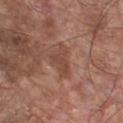This lesion was catalogued during total-body skin photography and was not selected for biopsy. From the chest. Measured at roughly 4 mm in maximum diameter. An algorithmic analysis of the crop reported an average lesion color of about L≈46 a*≈22 b*≈27 (CIELAB), a lesion–skin lightness drop of about 7, and a normalized border contrast of about 6. It also reported an automated nevus-likeness rating near 0 out of 100 and lesion-presence confidence of about 95/100. The tile uses white-light illumination. A close-up tile cropped from a whole-body skin photograph, about 15 mm across. A male patient, aged 63–67.